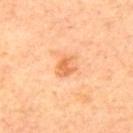| feature | finding |
|---|---|
| workup | no biopsy performed (imaged during a skin exam) |
| subject | male, in their mid-60s |
| acquisition | ~15 mm crop, total-body skin-cancer survey |
| lesion diameter | about 2.5 mm |
| illumination | cross-polarized illumination |
| site | the upper back |
| automated lesion analysis | an area of roughly 4.5 mm², an outline eccentricity of about 0.55 (0 = round, 1 = elongated), and a symmetry-axis asymmetry near 0.25; a mean CIELAB color near L≈67 a*≈28 b*≈44, about 10 CIELAB-L* units darker than the surrounding skin, and a lesion-to-skin contrast of about 6.5 (normalized; higher = more distinct); lesion-presence confidence of about 100/100 |